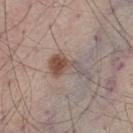Clinical impression: The lesion was photographed on a routine skin check and not biopsied; there is no pathology result. Background: From the left thigh. Automated tile analysis of the lesion measured peripheral color asymmetry of about 1. The software also gave lesion-presence confidence of about 95/100. This image is a 15 mm lesion crop taken from a total-body photograph. A male subject aged 43–47. About 5.5 mm across. Captured under white-light illumination.